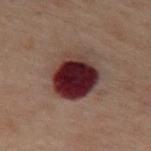Part of a total-body skin-imaging series; this lesion was reviewed on a skin check and was not flagged for biopsy. A male subject aged 58–62. From the mid back. The lesion's longest dimension is about 6 mm. A 15 mm close-up tile from a total-body photography series done for melanoma screening. Captured under cross-polarized illumination.